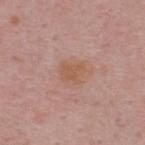notes = imaged on a skin check; not biopsied | diameter = about 3.5 mm | site = the upper back | subject = male, about 50 years old | image source = 15 mm crop, total-body photography | illumination = white-light illumination.